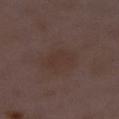notes: total-body-photography surveillance lesion; no biopsy | size: about 4 mm | tile lighting: white-light | location: the right lower leg | image-analysis metrics: a lesion color around L≈33 a*≈15 b*≈21 in CIELAB and about 4 CIELAB-L* units darker than the surrounding skin; a border-irregularity rating of about 3/10 and a peripheral color-asymmetry measure near 0.5 | subject: female, aged approximately 30 | acquisition: ~15 mm crop, total-body skin-cancer survey.